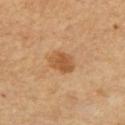biopsy status=catalogued during a skin exam; not biopsied | TBP lesion metrics=an average lesion color of about L≈52 a*≈21 b*≈38 (CIELAB) and a normalized lesion–skin contrast near 7.5; a nevus-likeness score of about 85/100 | size=~3 mm (longest diameter) | location=the arm | subject=male, aged 53 to 57 | acquisition=~15 mm tile from a whole-body skin photo.